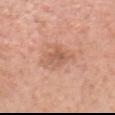Part of a total-body skin-imaging series; this lesion was reviewed on a skin check and was not flagged for biopsy.
A female subject, aged around 55.
A 15 mm close-up tile from a total-body photography series done for melanoma screening.
Captured under white-light illumination.
The lesion is on the head or neck.